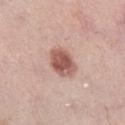The lesion's longest dimension is about 4 mm.
A female patient about 65 years old.
This is a white-light tile.
A 15 mm close-up tile from a total-body photography series done for melanoma screening.
The lesion is on the right lower leg.
An algorithmic analysis of the crop reported about 14 CIELAB-L* units darker than the surrounding skin and a normalized border contrast of about 9.5.